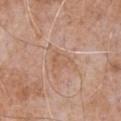Part of a total-body skin-imaging series; this lesion was reviewed on a skin check and was not flagged for biopsy. The subject is a male aged around 65. Captured under white-light illumination. A region of skin cropped from a whole-body photographic capture, roughly 15 mm wide. The lesion is on the chest. Approximately 2.5 mm at its widest.